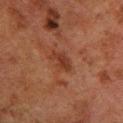  biopsy_status: not biopsied; imaged during a skin examination
  site: left lower leg
  lighting: cross-polarized
  lesion_size:
    long_diameter_mm_approx: 3.0
  patient:
    sex: male
    age_approx: 80
  image:
    source: total-body photography crop
    field_of_view_mm: 15